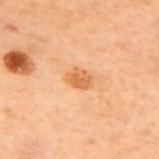Captured during whole-body skin photography for melanoma surveillance; the lesion was not biopsied. The lesion is on the upper back. A male subject, approximately 70 years of age. A 15 mm close-up extracted from a 3D total-body photography capture.